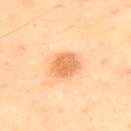Findings:
- workup — total-body-photography surveillance lesion; no biopsy
- image — 15 mm crop, total-body photography
- illumination — cross-polarized illumination
- diameter — ≈3.5 mm
- body site — the back
- subject — male, about 70 years old